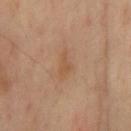Imaged during a routine full-body skin examination; the lesion was not biopsied and no histopathology is available. Longest diameter approximately 4 mm. A male subject, aged 63 to 67. A region of skin cropped from a whole-body photographic capture, roughly 15 mm wide. The total-body-photography lesion software estimated a shape eccentricity near 0.9 and a shape-asymmetry score of about 0.4 (0 = symmetric). It also reported an automated nevus-likeness rating near 0 out of 100. On the mid back.